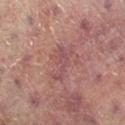Q: Was this lesion biopsied?
A: imaged on a skin check; not biopsied
Q: What did automated image analysis measure?
A: a lesion color around L≈44 a*≈22 b*≈18 in CIELAB, roughly 6 lightness units darker than nearby skin, and a normalized border contrast of about 5; a border-irregularity index near 6.5/10 and peripheral color asymmetry of about 0.5
Q: What lighting was used for the tile?
A: cross-polarized
Q: Lesion location?
A: the left leg
Q: What are the patient's age and sex?
A: female, aged approximately 80
Q: What kind of image is this?
A: ~15 mm crop, total-body skin-cancer survey
Q: Lesion size?
A: ≈4 mm